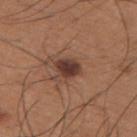| feature | finding |
|---|---|
| notes | catalogued during a skin exam; not biopsied |
| imaging modality | total-body-photography crop, ~15 mm field of view |
| image-analysis metrics | a mean CIELAB color near L≈37 a*≈20 b*≈24; a detector confidence of about 100 out of 100 that the crop contains a lesion |
| body site | the upper back |
| patient | male, aged around 35 |
| tile lighting | white-light illumination |